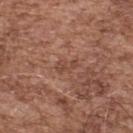Clinical impression: The lesion was tiled from a total-body skin photograph and was not biopsied.